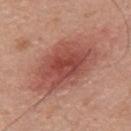  biopsy_status: not biopsied; imaged during a skin examination
  site: mid back
  image:
    source: total-body photography crop
    field_of_view_mm: 15
  patient:
    sex: male
    age_approx: 55
  lighting: white-light